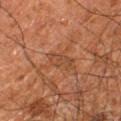  biopsy_status: not biopsied; imaged during a skin examination
  image:
    source: total-body photography crop
    field_of_view_mm: 15
  lesion_size:
    long_diameter_mm_approx: 3.5
  lighting: cross-polarized
  site: right leg
  patient:
    sex: male
    age_approx: 60
  automated_metrics:
    cielab_L: 44
    cielab_a: 23
    cielab_b: 33
    vs_skin_darker_L: 6.0
    nevus_likeness_0_100: 0
    lesion_detection_confidence_0_100: 100